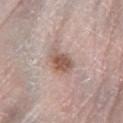Impression: Captured during whole-body skin photography for melanoma surveillance; the lesion was not biopsied. Clinical summary: From the leg. The lesion-visualizer software estimated a footprint of about 6.5 mm², an outline eccentricity of about 0.75 (0 = round, 1 = elongated), and a symmetry-axis asymmetry near 0.25. And it measured a lesion-detection confidence of about 100/100. A female subject, aged 63 to 67. Measured at roughly 3.5 mm in maximum diameter. Captured under white-light illumination. A 15 mm close-up extracted from a 3D total-body photography capture.